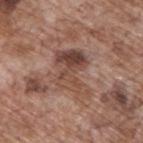follow-up: total-body-photography surveillance lesion; no biopsy | TBP lesion metrics: a mean CIELAB color near L≈46 a*≈19 b*≈25, roughly 10 lightness units darker than nearby skin, and a lesion-to-skin contrast of about 7.5 (normalized; higher = more distinct); border irregularity of about 5 on a 0–10 scale, a within-lesion color-variation index near 8.5/10, and a peripheral color-asymmetry measure near 3; a classifier nevus-likeness of about 0/100 and lesion-presence confidence of about 95/100 | illumination: white-light | patient: male, aged 68 to 72 | body site: the upper back | acquisition: ~15 mm crop, total-body skin-cancer survey | lesion size: ≈5.5 mm.